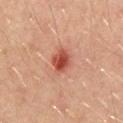No biopsy was performed on this lesion — it was imaged during a full skin examination and was not determined to be concerning. Measured at roughly 3 mm in maximum diameter. The subject is a male approximately 45 years of age. Captured under cross-polarized illumination. A region of skin cropped from a whole-body photographic capture, roughly 15 mm wide. From the chest. The lesion-visualizer software estimated about 11 CIELAB-L* units darker than the surrounding skin and a normalized lesion–skin contrast near 9. It also reported a border-irregularity index near 2/10 and a within-lesion color-variation index near 5/10.